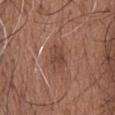  biopsy_status: not biopsied; imaged during a skin examination
  lesion_size:
    long_diameter_mm_approx: 3.5
  automated_metrics:
    area_mm2_approx: 6.0
    eccentricity: 0.7
    shape_asymmetry: 0.2
    border_irregularity_0_10: 2.5
    color_variation_0_10: 3.0
    peripheral_color_asymmetry: 1.0
  image:
    source: total-body photography crop
    field_of_view_mm: 15
  patient:
    sex: male
    age_approx: 45
  lighting: white-light
  site: head or neck